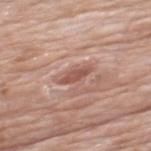biopsy status: total-body-photography surveillance lesion; no biopsy
illumination: white-light
image source: ~15 mm crop, total-body skin-cancer survey
location: the mid back
patient: male, aged 78–82